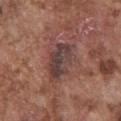The total-body-photography lesion software estimated an area of roughly 14 mm² and a symmetry-axis asymmetry near 0.3. And it measured a mean CIELAB color near L≈41 a*≈19 b*≈20 and a lesion–skin lightness drop of about 9. The software also gave a border-irregularity index near 4/10 and peripheral color asymmetry of about 2.
A roughly 15 mm field-of-view crop from a total-body skin photograph.
Located on the front of the torso.
About 5 mm across.
A male patient roughly 75 years of age.